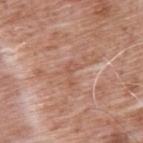Imaged during a routine full-body skin examination; the lesion was not biopsied and no histopathology is available. A male patient aged 58 to 62. On the back. This image is a 15 mm lesion crop taken from a total-body photograph. The tile uses white-light illumination.